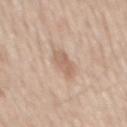notes=total-body-photography surveillance lesion; no biopsy
diameter=≈3.5 mm
subject=male, roughly 60 years of age
anatomic site=the mid back
image=~15 mm crop, total-body skin-cancer survey